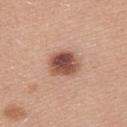A male subject aged 23–27. On the left upper arm. A region of skin cropped from a whole-body photographic capture, roughly 15 mm wide. The lesion-visualizer software estimated an eccentricity of roughly 0.6 and two-axis asymmetry of about 0.1. And it measured an average lesion color of about L≈51 a*≈23 b*≈28 (CIELAB). And it measured a border-irregularity rating of about 1/10, a color-variation rating of about 6/10, and a peripheral color-asymmetry measure near 2. The software also gave a detector confidence of about 100 out of 100 that the crop contains a lesion. This is a white-light tile. About 3.5 mm across.